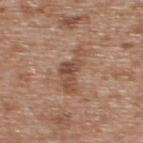Q: What is the anatomic site?
A: the upper back
Q: What is the lesion's diameter?
A: ~5.5 mm (longest diameter)
Q: What did automated image analysis measure?
A: a footprint of about 9 mm², an outline eccentricity of about 0.9 (0 = round, 1 = elongated), and a shape-asymmetry score of about 0.5 (0 = symmetric); a lesion–skin lightness drop of about 9; a color-variation rating of about 4/10 and a peripheral color-asymmetry measure near 1
Q: Patient demographics?
A: male, aged approximately 65
Q: How was this image acquired?
A: 15 mm crop, total-body photography